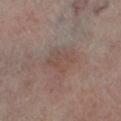Longest diameter approximately 3.5 mm.
A male patient, aged around 70.
Imaged with cross-polarized lighting.
A 15 mm close-up extracted from a 3D total-body photography capture.
Located on the left lower leg.